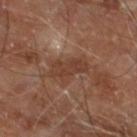Part of a total-body skin-imaging series; this lesion was reviewed on a skin check and was not flagged for biopsy. From the left lower leg. The subject is a male about 70 years old. A 15 mm crop from a total-body photograph taken for skin-cancer surveillance. The lesion's longest dimension is about 4.5 mm.